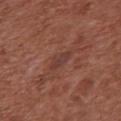Part of a total-body skin-imaging series; this lesion was reviewed on a skin check and was not flagged for biopsy. Approximately 3 mm at its widest. This image is a 15 mm lesion crop taken from a total-body photograph. The lesion is located on the front of the torso. Automated tile analysis of the lesion measured a mean CIELAB color near L≈38 a*≈22 b*≈24 and about 6 CIELAB-L* units darker than the surrounding skin. And it measured a border-irregularity index near 2.5/10 and internal color variation of about 1.5 on a 0–10 scale. The analysis additionally found a classifier nevus-likeness of about 0/100. A female subject, aged 73 to 77.